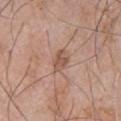<case>
  <biopsy_status>not biopsied; imaged during a skin examination</biopsy_status>
  <site>chest</site>
  <patient>
    <sex>male</sex>
    <age_approx>60</age_approx>
  </patient>
  <image>
    <source>total-body photography crop</source>
    <field_of_view_mm>15</field_of_view_mm>
  </image>
</case>